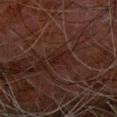biopsy status: total-body-photography surveillance lesion; no biopsy | acquisition: 15 mm crop, total-body photography | patient: male, approximately 80 years of age | body site: the arm | lesion size: ≈3 mm | automated metrics: a footprint of about 4 mm², an outline eccentricity of about 0.75 (0 = round, 1 = elongated), and two-axis asymmetry of about 0.3; an average lesion color of about L≈15 a*≈15 b*≈16 (CIELAB), roughly 4 lightness units darker than nearby skin, and a normalized lesion–skin contrast near 6 | lighting: cross-polarized.